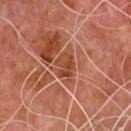notes: catalogued during a skin exam; not biopsied | lesion diameter: ≈3 mm | body site: the chest | imaging modality: total-body-photography crop, ~15 mm field of view | image-analysis metrics: an area of roughly 5.5 mm², an outline eccentricity of about 0.7 (0 = round, 1 = elongated), and a symmetry-axis asymmetry near 0.3; a border-irregularity index near 3/10 and internal color variation of about 4 on a 0–10 scale | lighting: cross-polarized | patient: male, aged 63–67.